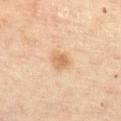Recorded during total-body skin imaging; not selected for excision or biopsy.
This image is a 15 mm lesion crop taken from a total-body photograph.
A female subject, aged around 45.
Approximately 2.5 mm at its widest.
The lesion is located on the front of the torso.
The total-body-photography lesion software estimated an area of roughly 5 mm², an eccentricity of roughly 0.5, and a shape-asymmetry score of about 0.2 (0 = symmetric). It also reported a lesion color around L≈65 a*≈19 b*≈37 in CIELAB, a lesion–skin lightness drop of about 9, and a lesion-to-skin contrast of about 6.5 (normalized; higher = more distinct). And it measured a classifier nevus-likeness of about 60/100 and lesion-presence confidence of about 100/100.
This is a cross-polarized tile.